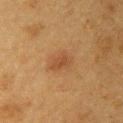Impression:
Captured during whole-body skin photography for melanoma surveillance; the lesion was not biopsied.
Acquisition and patient details:
The lesion is located on the right upper arm. The total-body-photography lesion software estimated border irregularity of about 2.5 on a 0–10 scale, a color-variation rating of about 2/10, and a peripheral color-asymmetry measure near 0.5. The analysis additionally found a nevus-likeness score of about 50/100 and lesion-presence confidence of about 100/100. A close-up tile cropped from a whole-body skin photograph, about 15 mm across. A female subject, approximately 40 years of age. This is a cross-polarized tile.